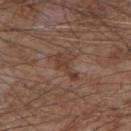* biopsy status — total-body-photography surveillance lesion; no biopsy
* automated metrics — a lesion area of about 4 mm² and an eccentricity of roughly 0.95; a lesion-detection confidence of about 100/100
* size — ~4 mm (longest diameter)
* lighting — white-light
* location — the right forearm
* acquisition — total-body-photography crop, ~15 mm field of view
* subject — male, roughly 75 years of age